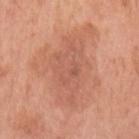Captured during whole-body skin photography for melanoma surveillance; the lesion was not biopsied. A region of skin cropped from a whole-body photographic capture, roughly 15 mm wide. The lesion is located on the left upper arm. The patient is a female aged around 65.